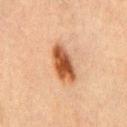biopsy status=total-body-photography surveillance lesion; no biopsy | image source=~15 mm crop, total-body skin-cancer survey | lesion diameter=~5 mm (longest diameter) | subject=male, approximately 70 years of age | illumination=cross-polarized | site=the front of the torso.